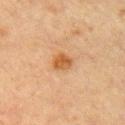illumination: cross-polarized
lesion diameter: ~3 mm (longest diameter)
anatomic site: the left upper arm
image source: ~15 mm tile from a whole-body skin photo
patient: male, aged approximately 65